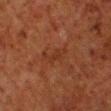follow-up=catalogued during a skin exam; not biopsied | TBP lesion metrics=an area of roughly 4 mm², a shape eccentricity near 0.85, and a symmetry-axis asymmetry near 0.55 | image=15 mm crop, total-body photography | lesion size=~3 mm (longest diameter) | location=the left lower leg | subject=male, aged 78 to 82.